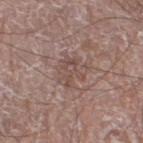workup: no biopsy performed (imaged during a skin exam)
site: the left lower leg
TBP lesion metrics: a footprint of about 8.5 mm² and a shape-asymmetry score of about 0.35 (0 = symmetric); roughly 7 lightness units darker than nearby skin and a normalized border contrast of about 5
patient: male, in their mid-70s
acquisition: ~15 mm tile from a whole-body skin photo